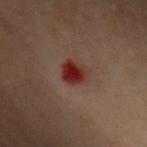Findings:
- follow-up: imaged on a skin check; not biopsied
- illumination: cross-polarized
- image source: ~15 mm tile from a whole-body skin photo
- subject: female, approximately 60 years of age
- image-analysis metrics: an area of roughly 5.5 mm², an outline eccentricity of about 0.6 (0 = round, 1 = elongated), and a symmetry-axis asymmetry near 0.2; an average lesion color of about L≈25 a*≈25 b*≈22 (CIELAB) and a normalized lesion–skin contrast near 11.5; a border-irregularity rating of about 2/10, a color-variation rating of about 4/10, and radial color variation of about 1.5
- lesion size: ≈3 mm
- site: the right arm